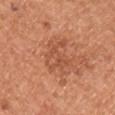This lesion was catalogued during total-body skin photography and was not selected for biopsy.
A male subject, approximately 55 years of age.
From the chest.
The total-body-photography lesion software estimated a lesion area of about 8.5 mm², a shape eccentricity near 0.7, and two-axis asymmetry of about 0.55. The analysis additionally found border irregularity of about 7.5 on a 0–10 scale, a within-lesion color-variation index near 4.5/10, and peripheral color asymmetry of about 2. And it measured a classifier nevus-likeness of about 30/100 and a detector confidence of about 100 out of 100 that the crop contains a lesion.
Captured under white-light illumination.
This image is a 15 mm lesion crop taken from a total-body photograph.
Approximately 4.5 mm at its widest.